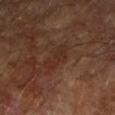Clinical summary: Approximately 3.5 mm at its widest. Automated tile analysis of the lesion measured an eccentricity of roughly 0.9. And it measured a lesion–skin lightness drop of about 5 and a normalized lesion–skin contrast near 5.5. And it measured border irregularity of about 5 on a 0–10 scale, a within-lesion color-variation index near 2/10, and peripheral color asymmetry of about 0.5. And it measured an automated nevus-likeness rating near 0 out of 100 and a lesion-detection confidence of about 100/100. This is a cross-polarized tile. A male subject about 65 years old. A 15 mm close-up tile from a total-body photography series done for melanoma screening. On the right forearm.